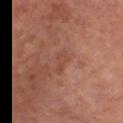Impression: The lesion was photographed on a routine skin check and not biopsied; there is no pathology result. Context: A 15 mm crop from a total-body photograph taken for skin-cancer surveillance. The subject is a female aged 48–52. On the left thigh.